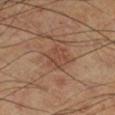| field | value |
|---|---|
| image-analysis metrics | a lesion color around L≈44 a*≈20 b*≈28 in CIELAB, about 6 CIELAB-L* units darker than the surrounding skin, and a normalized border contrast of about 5 |
| image | total-body-photography crop, ~15 mm field of view |
| tile lighting | cross-polarized illumination |
| body site | the right lower leg |
| lesion size | ≈3.5 mm |
| subject | male, about 70 years old |